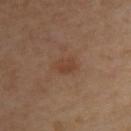No biopsy was performed on this lesion — it was imaged during a full skin examination and was not determined to be concerning. A region of skin cropped from a whole-body photographic capture, roughly 15 mm wide. A female subject approximately 45 years of age. On the upper back. This is a cross-polarized tile.